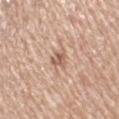No biopsy was performed on this lesion — it was imaged during a full skin examination and was not determined to be concerning.
An algorithmic analysis of the crop reported a lesion color around L≈60 a*≈19 b*≈28 in CIELAB and a lesion-to-skin contrast of about 7 (normalized; higher = more distinct). It also reported an automated nevus-likeness rating near 0 out of 100 and a lesion-detection confidence of about 100/100.
A 15 mm close-up extracted from a 3D total-body photography capture.
Measured at roughly 2.5 mm in maximum diameter.
The tile uses white-light illumination.
The lesion is located on the arm.
The subject is a female in their 70s.